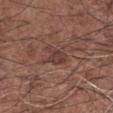Assessment: The lesion was tiled from a total-body skin photograph and was not biopsied. Clinical summary: The patient is a male aged approximately 75. Imaged with white-light lighting. The lesion is located on the right forearm. A lesion tile, about 15 mm wide, cut from a 3D total-body photograph. Longest diameter approximately 3 mm.